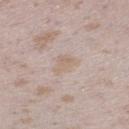notes — catalogued during a skin exam; not biopsied
image source — ~15 mm crop, total-body skin-cancer survey
illumination — white-light illumination
anatomic site — the right lower leg
subject — female, aged approximately 25
automated metrics — a lesion–skin lightness drop of about 5; a border-irregularity index near 3.5/10, a color-variation rating of about 2.5/10, and a peripheral color-asymmetry measure near 1